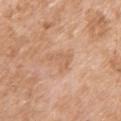Captured during whole-body skin photography for melanoma surveillance; the lesion was not biopsied.
On the right upper arm.
The patient is a female about 75 years old.
A roughly 15 mm field-of-view crop from a total-body skin photograph.